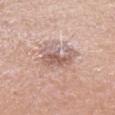biopsy status: no biopsy performed (imaged during a skin exam)
site: the head or neck
TBP lesion metrics: a footprint of about 12 mm², an eccentricity of roughly 0.55, and two-axis asymmetry of about 0.25; an automated nevus-likeness rating near 0 out of 100 and a lesion-detection confidence of about 100/100
image source: 15 mm crop, total-body photography
lesion diameter: about 4.5 mm
patient: female, aged approximately 65
illumination: white-light